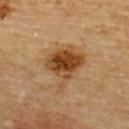Q: Is there a histopathology result?
A: catalogued during a skin exam; not biopsied
Q: What is the lesion's diameter?
A: ≈5.5 mm
Q: What did automated image analysis measure?
A: a lesion color around L≈44 a*≈21 b*≈37 in CIELAB, about 13 CIELAB-L* units darker than the surrounding skin, and a normalized lesion–skin contrast near 10
Q: What is the anatomic site?
A: the upper back
Q: How was this image acquired?
A: ~15 mm crop, total-body skin-cancer survey
Q: Who is the patient?
A: male, in their mid-80s or older
Q: How was the tile lit?
A: cross-polarized